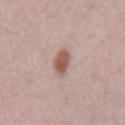This is a white-light tile. About 3 mm across. A female subject, roughly 45 years of age. This image is a 15 mm lesion crop taken from a total-body photograph. The lesion is on the right upper arm. Automated image analysis of the tile measured a mean CIELAB color near L≈56 a*≈20 b*≈25, about 12 CIELAB-L* units darker than the surrounding skin, and a normalized border contrast of about 8.5.